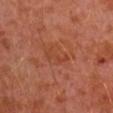Part of a total-body skin-imaging series; this lesion was reviewed on a skin check and was not flagged for biopsy. The patient is a male aged 28 to 32. This is a cross-polarized tile. This image is a 15 mm lesion crop taken from a total-body photograph. On the left arm.